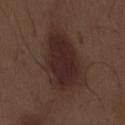follow-up: catalogued during a skin exam; not biopsied
size: ≈7.5 mm
tile lighting: white-light illumination
acquisition: ~15 mm tile from a whole-body skin photo
site: the abdomen
automated metrics: a mean CIELAB color near L≈26 a*≈17 b*≈18, roughly 9 lightness units darker than nearby skin, and a normalized border contrast of about 10
subject: male, in their 70s